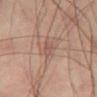Imaged during a routine full-body skin examination; the lesion was not biopsied and no histopathology is available.
A close-up tile cropped from a whole-body skin photograph, about 15 mm across.
The subject is a male about 40 years old.
The lesion is on the left thigh.